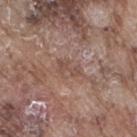Impression: Part of a total-body skin-imaging series; this lesion was reviewed on a skin check and was not flagged for biopsy. Image and clinical context: Located on the leg. Captured under white-light illumination. Longest diameter approximately 3 mm. A male patient approximately 75 years of age. A roughly 15 mm field-of-view crop from a total-body skin photograph.